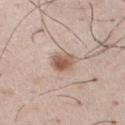biopsy status=catalogued during a skin exam; not biopsied
patient=male, aged around 50
lesion size=~3 mm (longest diameter)
tile lighting=white-light
anatomic site=the left thigh
acquisition=total-body-photography crop, ~15 mm field of view